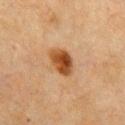Findings:
• follow-up: imaged on a skin check; not biopsied
• size: ≈4 mm
• patient: female, in their mid- to late 50s
• image: ~15 mm crop, total-body skin-cancer survey
• site: the front of the torso